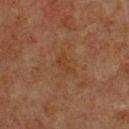* notes: total-body-photography surveillance lesion; no biopsy
* TBP lesion metrics: an area of roughly 4 mm², a shape eccentricity near 0.85, and a symmetry-axis asymmetry near 0.5; a lesion color around L≈31 a*≈17 b*≈27 in CIELAB, roughly 4 lightness units darker than nearby skin, and a normalized border contrast of about 4.5
* subject: male, approximately 75 years of age
* lesion size: ~3 mm (longest diameter)
* illumination: cross-polarized illumination
* anatomic site: the front of the torso
* image source: ~15 mm crop, total-body skin-cancer survey